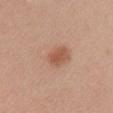workup: imaged on a skin check; not biopsied
body site: the right upper arm
imaging modality: total-body-photography crop, ~15 mm field of view
lighting: white-light
subject: female, in their mid- to late 30s